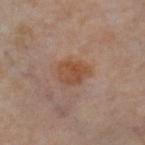Impression:
This lesion was catalogued during total-body skin photography and was not selected for biopsy.
Background:
A female patient, roughly 50 years of age. A roughly 15 mm field-of-view crop from a total-body skin photograph. The lesion is on the left thigh.